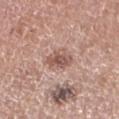{"biopsy_status": "not biopsied; imaged during a skin examination", "automated_metrics": {"cielab_L": 54, "cielab_a": 21, "cielab_b": 25, "vs_skin_contrast_norm": 7.5}, "site": "right forearm", "image": {"source": "total-body photography crop", "field_of_view_mm": 15}, "patient": {"sex": "female", "age_approx": 50}, "lesion_size": {"long_diameter_mm_approx": 3.0}}